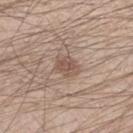{
  "biopsy_status": "not biopsied; imaged during a skin examination",
  "image": {
    "source": "total-body photography crop",
    "field_of_view_mm": 15
  },
  "patient": {
    "sex": "male",
    "age_approx": 30
  },
  "lighting": "white-light",
  "site": "arm",
  "automated_metrics": {
    "area_mm2_approx": 5.5,
    "eccentricity": 0.65,
    "shape_asymmetry": 0.2,
    "cielab_L": 53,
    "cielab_a": 16,
    "cielab_b": 25,
    "vs_skin_darker_L": 9.0,
    "vs_skin_contrast_norm": 6.5,
    "border_irregularity_0_10": 2.5,
    "color_variation_0_10": 2.5,
    "peripheral_color_asymmetry": 1.0,
    "nevus_likeness_0_100": 15,
    "lesion_detection_confidence_0_100": 100
  }
}